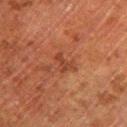This lesion was catalogued during total-body skin photography and was not selected for biopsy.
Captured under cross-polarized illumination.
Automated image analysis of the tile measured internal color variation of about 1.5 on a 0–10 scale. And it measured a classifier nevus-likeness of about 0/100 and a lesion-detection confidence of about 100/100.
The lesion is located on the left lower leg.
A 15 mm close-up tile from a total-body photography series done for melanoma screening.
A male subject about 80 years old.
Approximately 3 mm at its widest.